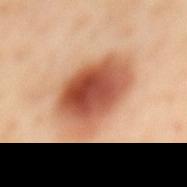Q: Is there a histopathology result?
A: imaged on a skin check; not biopsied
Q: Illumination type?
A: cross-polarized illumination
Q: Lesion location?
A: the mid back
Q: What are the patient's age and sex?
A: female, aged around 55
Q: What did automated image analysis measure?
A: border irregularity of about 2 on a 0–10 scale, a color-variation rating of about 9.5/10, and peripheral color asymmetry of about 3
Q: How was this image acquired?
A: ~15 mm crop, total-body skin-cancer survey